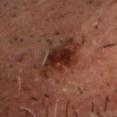| field | value |
|---|---|
| lesion diameter | ~6.5 mm (longest diameter) |
| image | 15 mm crop, total-body photography |
| patient | male, aged 48 to 52 |
| anatomic site | the head or neck |
| illumination | cross-polarized |